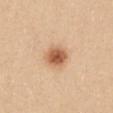This lesion was catalogued during total-body skin photography and was not selected for biopsy. A female patient, roughly 25 years of age. This image is a 15 mm lesion crop taken from a total-body photograph. The lesion is on the mid back. Imaged with white-light lighting. Measured at roughly 3.5 mm in maximum diameter.